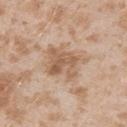Recorded during total-body skin imaging; not selected for excision or biopsy. Longest diameter approximately 4.5 mm. Automated tile analysis of the lesion measured a lesion area of about 13 mm², an eccentricity of roughly 0.55, and a symmetry-axis asymmetry near 0.2. The analysis additionally found a border-irregularity rating of about 3/10, internal color variation of about 5 on a 0–10 scale, and radial color variation of about 2. The analysis additionally found a classifier nevus-likeness of about 0/100 and lesion-presence confidence of about 100/100. A 15 mm close-up extracted from a 3D total-body photography capture. The tile uses white-light illumination. From the left upper arm. A female patient roughly 25 years of age.